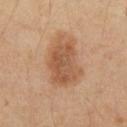Assessment: Captured during whole-body skin photography for melanoma surveillance; the lesion was not biopsied. Context: This is a cross-polarized tile. The patient is a male aged approximately 65. From the chest. About 6 mm across. Cropped from a total-body skin-imaging series; the visible field is about 15 mm.